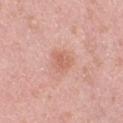Imaged during a routine full-body skin examination; the lesion was not biopsied and no histopathology is available. An algorithmic analysis of the crop reported a lesion–skin lightness drop of about 8 and a normalized lesion–skin contrast near 5.5. The software also gave a border-irregularity index near 2.5/10, a color-variation rating of about 2/10, and peripheral color asymmetry of about 0.5. A lesion tile, about 15 mm wide, cut from a 3D total-body photograph. Longest diameter approximately 3 mm. Imaged with white-light lighting. The patient is a female approximately 40 years of age. Located on the right thigh.